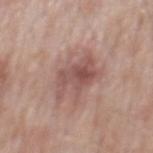Recorded during total-body skin imaging; not selected for excision or biopsy.
Automated tile analysis of the lesion measured an area of roughly 11 mm², an eccentricity of roughly 0.65, and two-axis asymmetry of about 0.45.
Cropped from a total-body skin-imaging series; the visible field is about 15 mm.
On the mid back.
A male subject aged 73 to 77.